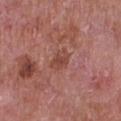Image and clinical context:
The tile uses white-light illumination. A male subject, in their mid-60s. A lesion tile, about 15 mm wide, cut from a 3D total-body photograph. The lesion is on the front of the torso. Measured at roughly 3 mm in maximum diameter.